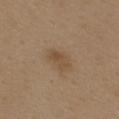Part of a total-body skin-imaging series; this lesion was reviewed on a skin check and was not flagged for biopsy.
On the back.
Cropped from a whole-body photographic skin survey; the tile spans about 15 mm.
The patient is a female aged approximately 40.
Captured under white-light illumination.
Approximately 3 mm at its widest.